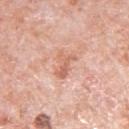Clinical impression: Part of a total-body skin-imaging series; this lesion was reviewed on a skin check and was not flagged for biopsy. Image and clinical context: A male subject, about 80 years old. A lesion tile, about 15 mm wide, cut from a 3D total-body photograph. On the left upper arm.